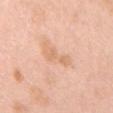| feature | finding |
|---|---|
| biopsy status | imaged on a skin check; not biopsied |
| patient | female, aged approximately 40 |
| body site | the chest |
| lesion size | ~4 mm (longest diameter) |
| image-analysis metrics | an area of roughly 5.5 mm², a shape eccentricity near 0.95, and two-axis asymmetry of about 0.4; a lesion–skin lightness drop of about 7 and a lesion-to-skin contrast of about 5.5 (normalized; higher = more distinct); a border-irregularity rating of about 5.5/10 and a color-variation rating of about 1.5/10 |
| image | total-body-photography crop, ~15 mm field of view |